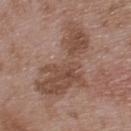biopsy_status: not biopsied; imaged during a skin examination
lesion_size:
  long_diameter_mm_approx: 9.0
lighting: white-light
patient:
  sex: male
  age_approx: 50
site: upper back
image:
  source: total-body photography crop
  field_of_view_mm: 15